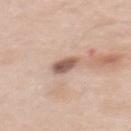Clinical impression:
This lesion was catalogued during total-body skin photography and was not selected for biopsy.
Acquisition and patient details:
The lesion is on the mid back. Captured under white-light illumination. A male subject roughly 60 years of age. Approximately 2.5 mm at its widest. A 15 mm close-up tile from a total-body photography series done for melanoma screening.